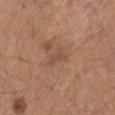A male patient, aged 73 to 77. Located on the mid back. A lesion tile, about 15 mm wide, cut from a 3D total-body photograph.